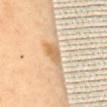| key | value |
|---|---|
| biopsy status | no biopsy performed (imaged during a skin exam) |
| body site | the mid back |
| patient | female, in their mid- to late 50s |
| lesion size | ≈3.5 mm |
| image source | 15 mm crop, total-body photography |
| illumination | cross-polarized illumination |
| automated metrics | an area of roughly 5.5 mm²; internal color variation of about 3.5 on a 0–10 scale and a peripheral color-asymmetry measure near 1.5; an automated nevus-likeness rating near 55 out of 100 |